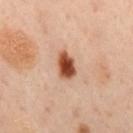follow-up: total-body-photography surveillance lesion; no biopsy | tile lighting: cross-polarized | lesion size: ~3.5 mm (longest diameter) | image-analysis metrics: a border-irregularity index near 2/10, internal color variation of about 3.5 on a 0–10 scale, and a peripheral color-asymmetry measure near 0.5; lesion-presence confidence of about 100/100 | site: the mid back | image source: 15 mm crop, total-body photography | subject: male, aged around 50.